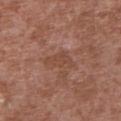biopsy status = total-body-photography surveillance lesion; no biopsy
imaging modality = 15 mm crop, total-body photography
subject = male, aged approximately 45
anatomic site = the chest